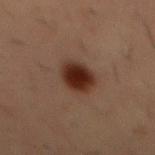Findings:
* image source · total-body-photography crop, ~15 mm field of view
* lighting · cross-polarized illumination
* lesion size · about 4 mm
* anatomic site · the abdomen
* patient · male, aged 53 to 57